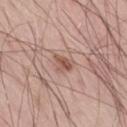automated metrics: a lesion area of about 4 mm², an outline eccentricity of about 0.55 (0 = round, 1 = elongated), and a symmetry-axis asymmetry near 0.35; a classifier nevus-likeness of about 50/100 and a lesion-detection confidence of about 100/100 | imaging modality: ~15 mm crop, total-body skin-cancer survey | subject: male, aged approximately 55 | lesion diameter: ≈2.5 mm | body site: the right thigh.